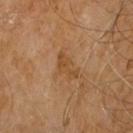The lesion was tiled from a total-body skin photograph and was not biopsied. A lesion tile, about 15 mm wide, cut from a 3D total-body photograph. The patient is a male aged around 60. The lesion is located on the chest.